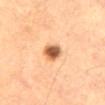site — the lower back | subject — male, roughly 65 years of age | acquisition — ~15 mm crop, total-body skin-cancer survey.